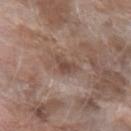biopsy status: imaged on a skin check; not biopsied
site: the left forearm
image source: total-body-photography crop, ~15 mm field of view
subject: female, aged around 75